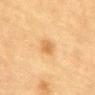Recorded during total-body skin imaging; not selected for excision or biopsy. The lesion is located on the mid back. The patient is a female in their 80s. A close-up tile cropped from a whole-body skin photograph, about 15 mm across. Measured at roughly 2.5 mm in maximum diameter.